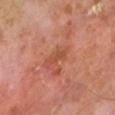biopsy status=catalogued during a skin exam; not biopsied
image=total-body-photography crop, ~15 mm field of view
patient=male, aged around 65